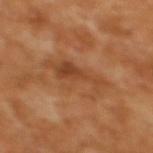The lesion was photographed on a routine skin check and not biopsied; there is no pathology result. On the back. A female subject, in their mid- to late 50s. A close-up tile cropped from a whole-body skin photograph, about 15 mm across.